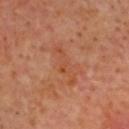Assessment: Recorded during total-body skin imaging; not selected for excision or biopsy. Acquisition and patient details: From the head or neck. A lesion tile, about 15 mm wide, cut from a 3D total-body photograph. A male subject, approximately 65 years of age. Automated tile analysis of the lesion measured a footprint of about 6 mm², a shape eccentricity near 0.95, and a symmetry-axis asymmetry near 0.45. It also reported a lesion-to-skin contrast of about 5 (normalized; higher = more distinct). Longest diameter approximately 5 mm. Captured under cross-polarized illumination.